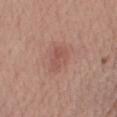* workup · catalogued during a skin exam; not biopsied
* acquisition · total-body-photography crop, ~15 mm field of view
* patient · male, aged around 60
* lesion diameter · ≈4 mm
* anatomic site · the arm